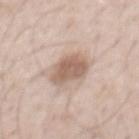Captured during whole-body skin photography for melanoma surveillance; the lesion was not biopsied.
The lesion's longest dimension is about 4.5 mm.
A region of skin cropped from a whole-body photographic capture, roughly 15 mm wide.
Imaged with white-light lighting.
The subject is a male roughly 40 years of age.
The lesion is on the mid back.